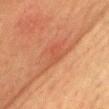Q: Was a biopsy performed?
A: total-body-photography surveillance lesion; no biopsy
Q: Lesion location?
A: the head or neck
Q: Who is the patient?
A: female, in their mid- to late 50s
Q: How was this image acquired?
A: 15 mm crop, total-body photography
Q: What lighting was used for the tile?
A: cross-polarized
Q: What is the lesion's diameter?
A: ≈7 mm